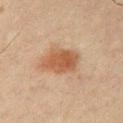| feature | finding |
|---|---|
| biopsy status | catalogued during a skin exam; not biopsied |
| site | the chest |
| size | ≈4 mm |
| image source | ~15 mm crop, total-body skin-cancer survey |
| subject | male, aged 58 to 62 |
| tile lighting | cross-polarized illumination |